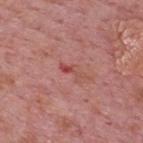notes: total-body-photography surveillance lesion; no biopsy | automated lesion analysis: an area of roughly 2.5 mm² and a shape eccentricity near 0.95; border irregularity of about 6.5 on a 0–10 scale, a within-lesion color-variation index near 0/10, and a peripheral color-asymmetry measure near 0; an automated nevus-likeness rating near 0 out of 100 and lesion-presence confidence of about 95/100 | illumination: white-light illumination | imaging modality: ~15 mm tile from a whole-body skin photo | location: the back | patient: male, in their mid- to late 70s.